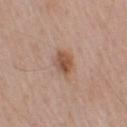<tbp_lesion>
  <biopsy_status>not biopsied; imaged during a skin examination</biopsy_status>
  <automated_metrics>
    <border_irregularity_0_10>2.0</border_irregularity_0_10>
    <color_variation_0_10>5.0</color_variation_0_10>
  </automated_metrics>
  <site>left upper arm</site>
  <lesion_size>
    <long_diameter_mm_approx>3.5</long_diameter_mm_approx>
  </lesion_size>
  <image>
    <source>total-body photography crop</source>
    <field_of_view_mm>15</field_of_view_mm>
  </image>
  <patient>
    <sex>male</sex>
    <age_approx>65</age_approx>
  </patient>
</tbp_lesion>